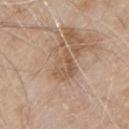acquisition: ~15 mm crop, total-body skin-cancer survey
lesion diameter: about 5 mm
TBP lesion metrics: an average lesion color of about L≈56 a*≈17 b*≈31 (CIELAB), a lesion–skin lightness drop of about 7, and a normalized border contrast of about 5.5; border irregularity of about 5 on a 0–10 scale, internal color variation of about 4.5 on a 0–10 scale, and radial color variation of about 1.5; a nevus-likeness score of about 0/100 and a lesion-detection confidence of about 100/100
body site: the chest
patient: male, aged approximately 80
illumination: white-light